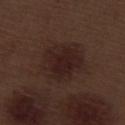Assessment: Recorded during total-body skin imaging; not selected for excision or biopsy. Background: A 15 mm close-up tile from a total-body photography series done for melanoma screening. From the right lower leg. The patient is a male about 70 years old.